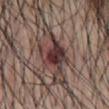Notes:
• workup: catalogued during a skin exam; not biopsied
• image-analysis metrics: a lesion area of about 10 mm², a shape eccentricity near 0.65, and a shape-asymmetry score of about 0.45 (0 = symmetric); border irregularity of about 5.5 on a 0–10 scale, internal color variation of about 8 on a 0–10 scale, and radial color variation of about 2.5; a lesion-detection confidence of about 100/100
• anatomic site: the abdomen
• tile lighting: white-light
• image: ~15 mm crop, total-body skin-cancer survey
• size: about 5.5 mm
• subject: male, aged approximately 55